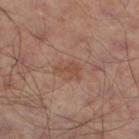notes: imaged on a skin check; not biopsied | image source: ~15 mm tile from a whole-body skin photo | automated lesion analysis: an area of roughly 2.5 mm², a shape eccentricity near 0.9, and a symmetry-axis asymmetry near 0.55; an average lesion color of about L≈46 a*≈21 b*≈28 (CIELAB) and a lesion–skin lightness drop of about 7; border irregularity of about 6 on a 0–10 scale, a color-variation rating of about 0/10, and peripheral color asymmetry of about 0; lesion-presence confidence of about 100/100 | body site: the left leg | subject: male, roughly 50 years of age | tile lighting: cross-polarized.